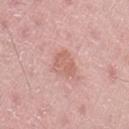Assessment: Recorded during total-body skin imaging; not selected for excision or biopsy.